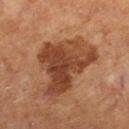Context:
Imaged with cross-polarized lighting. A roughly 15 mm field-of-view crop from a total-body skin photograph. About 8 mm across. A female subject, aged 63–67. The lesion is on the right lower leg.